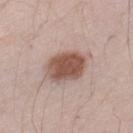Automated image analysis of the tile measured a footprint of about 16 mm², a shape eccentricity near 0.8, and a symmetry-axis asymmetry near 0.15. The software also gave a lesion color around L≈54 a*≈19 b*≈25 in CIELAB, roughly 15 lightness units darker than nearby skin, and a lesion-to-skin contrast of about 10 (normalized; higher = more distinct). The analysis additionally found a border-irregularity rating of about 2.5/10, a within-lesion color-variation index near 4.5/10, and a peripheral color-asymmetry measure near 1.5. It also reported a detector confidence of about 100 out of 100 that the crop contains a lesion.
The lesion is located on the left upper arm.
The lesion's longest dimension is about 6.5 mm.
A male patient, aged approximately 45.
Imaged with white-light lighting.
Cropped from a total-body skin-imaging series; the visible field is about 15 mm.